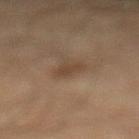Imaged during a routine full-body skin examination; the lesion was not biopsied and no histopathology is available. A male subject, in their mid-60s. Captured under cross-polarized illumination. A 15 mm close-up extracted from a 3D total-body photography capture. From the left lower leg. Measured at roughly 2.5 mm in maximum diameter.